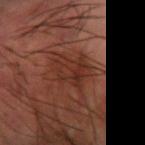* notes — no biopsy performed (imaged during a skin exam)
* diameter — ~5.5 mm (longest diameter)
* site — the right forearm
* subject — male, aged approximately 60
* acquisition — total-body-photography crop, ~15 mm field of view
* lighting — cross-polarized illumination